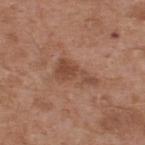Findings:
– follow-up: total-body-photography surveillance lesion; no biopsy
– lesion diameter: ~4.5 mm (longest diameter)
– acquisition: 15 mm crop, total-body photography
– illumination: white-light illumination
– subject: male, roughly 55 years of age
– automated lesion analysis: a border-irregularity index near 7/10 and a color-variation rating of about 2/10
– site: the upper back